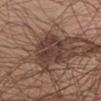biopsy status — no biopsy performed (imaged during a skin exam) | image source — ~15 mm tile from a whole-body skin photo | location — the chest | lesion size — ~5.5 mm (longest diameter) | image-analysis metrics — a footprint of about 14 mm², an outline eccentricity of about 0.8 (0 = round, 1 = elongated), and two-axis asymmetry of about 0.25; a nevus-likeness score of about 40/100 | subject — male, approximately 55 years of age.